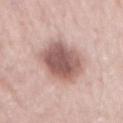Q: Is there a histopathology result?
A: no biopsy performed (imaged during a skin exam)